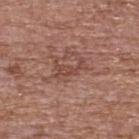image-analysis metrics — a border-irregularity index near 7.5/10, internal color variation of about 0 on a 0–10 scale, and radial color variation of about 0; a classifier nevus-likeness of about 0/100 and lesion-presence confidence of about 95/100
patient — female, aged around 65
image source — total-body-photography crop, ~15 mm field of view
location — the upper back